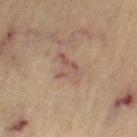Captured during whole-body skin photography for melanoma surveillance; the lesion was not biopsied. The lesion is located on the left thigh. About 3.5 mm across. A 15 mm close-up tile from a total-body photography series done for melanoma screening. This is a cross-polarized tile. The total-body-photography lesion software estimated an automated nevus-likeness rating near 0 out of 100 and a detector confidence of about 100 out of 100 that the crop contains a lesion. A female patient, aged around 65.